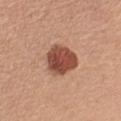Recorded during total-body skin imaging; not selected for excision or biopsy.
A close-up tile cropped from a whole-body skin photograph, about 15 mm across.
An algorithmic analysis of the crop reported border irregularity of about 2 on a 0–10 scale, internal color variation of about 4.5 on a 0–10 scale, and radial color variation of about 1.5. The software also gave a detector confidence of about 100 out of 100 that the crop contains a lesion.
A female subject, aged 53–57.
From the left upper arm.
Longest diameter approximately 4.5 mm.
The tile uses white-light illumination.